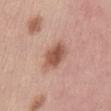<record>
  <biopsy_status>not biopsied; imaged during a skin examination</biopsy_status>
  <patient>
    <sex>female</sex>
    <age_approx>35</age_approx>
  </patient>
  <image>
    <source>total-body photography crop</source>
    <field_of_view_mm>15</field_of_view_mm>
  </image>
  <site>abdomen</site>
  <lighting>white-light</lighting>
</record>